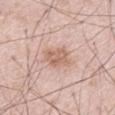The lesion-visualizer software estimated a border-irregularity index near 2/10, a within-lesion color-variation index near 2.5/10, and peripheral color asymmetry of about 1. The software also gave an automated nevus-likeness rating near 20 out of 100 and lesion-presence confidence of about 100/100. The lesion's longest dimension is about 3.5 mm. The tile uses white-light illumination. Located on the left thigh. This image is a 15 mm lesion crop taken from a total-body photograph. A male subject, aged around 55.